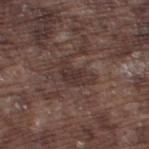workup = imaged on a skin check; not biopsied
tile lighting = white-light
imaging modality = 15 mm crop, total-body photography
subject = male, approximately 75 years of age
site = the left thigh
lesion diameter = ~4 mm (longest diameter)